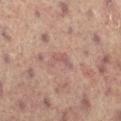follow-up — imaged on a skin check; not biopsied | subject — female, aged 78–82 | body site — the left leg | imaging modality — ~15 mm crop, total-body skin-cancer survey | lesion diameter — about 3 mm | lighting — cross-polarized.